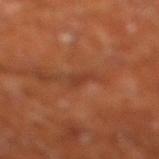Notes:
* workup · total-body-photography surveillance lesion; no biopsy
* TBP lesion metrics · an eccentricity of roughly 0.9 and a shape-asymmetry score of about 0.4 (0 = symmetric); a classifier nevus-likeness of about 0/100
* anatomic site · the right lower leg
* subject · male, aged 63 to 67
* image · ~15 mm crop, total-body skin-cancer survey
* size · about 3 mm